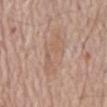Clinical impression: No biopsy was performed on this lesion — it was imaged during a full skin examination and was not determined to be concerning. Context: Located on the abdomen. A 15 mm close-up extracted from a 3D total-body photography capture. The subject is a male aged around 70.